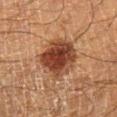follow-up = no biopsy performed (imaged during a skin exam); patient = male, aged 58 to 62; lesion diameter = ~5 mm (longest diameter); image source = ~15 mm crop, total-body skin-cancer survey; body site = the right lower leg; lighting = cross-polarized.